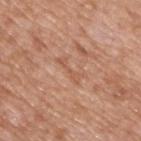{"biopsy_status": "not biopsied; imaged during a skin examination", "image": {"source": "total-body photography crop", "field_of_view_mm": 15}, "site": "upper back", "patient": {"sex": "male", "age_approx": 50}}